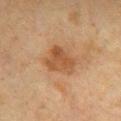Imaged during a routine full-body skin examination; the lesion was not biopsied and no histopathology is available. The recorded lesion diameter is about 4 mm. Automated tile analysis of the lesion measured an eccentricity of roughly 0.65 and a shape-asymmetry score of about 0.35 (0 = symmetric). The software also gave an average lesion color of about L≈42 a*≈19 b*≈31 (CIELAB), about 8 CIELAB-L* units darker than the surrounding skin, and a lesion-to-skin contrast of about 7 (normalized; higher = more distinct). A female patient, aged approximately 55. A region of skin cropped from a whole-body photographic capture, roughly 15 mm wide. This is a cross-polarized tile. Located on the chest.